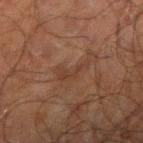Captured during whole-body skin photography for melanoma surveillance; the lesion was not biopsied. Captured under cross-polarized illumination. Measured at roughly 3.5 mm in maximum diameter. A male patient aged around 70. A 15 mm crop from a total-body photograph taken for skin-cancer surveillance. On the right upper arm.